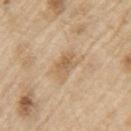Clinical impression: No biopsy was performed on this lesion — it was imaged during a full skin examination and was not determined to be concerning. Image and clinical context: A male subject aged around 70. A close-up tile cropped from a whole-body skin photograph, about 15 mm across. This is a white-light tile. Measured at roughly 3 mm in maximum diameter. On the left upper arm.